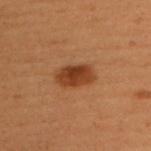Assessment:
The lesion was tiled from a total-body skin photograph and was not biopsied.
Image and clinical context:
Longest diameter approximately 4 mm. A female subject aged 48 to 52. A close-up tile cropped from a whole-body skin photograph, about 15 mm across. On the back. The total-body-photography lesion software estimated a footprint of about 8 mm², a shape eccentricity near 0.85, and two-axis asymmetry of about 0.2. The analysis additionally found a mean CIELAB color near L≈34 a*≈22 b*≈32 and a lesion–skin lightness drop of about 11. The analysis additionally found border irregularity of about 2 on a 0–10 scale, a color-variation rating of about 3.5/10, and radial color variation of about 1.